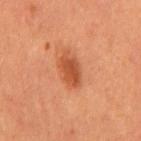– notes: imaged on a skin check; not biopsied
– lesion diameter: ≈4 mm
– subject: male, aged around 65
– location: the mid back
– image: total-body-photography crop, ~15 mm field of view